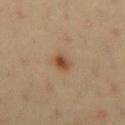No biopsy was performed on this lesion — it was imaged during a full skin examination and was not determined to be concerning. Imaged with cross-polarized lighting. A region of skin cropped from a whole-body photographic capture, roughly 15 mm wide. Automated image analysis of the tile measured a border-irregularity rating of about 2.5/10 and a peripheral color-asymmetry measure near 2. The software also gave lesion-presence confidence of about 100/100. About 2.5 mm across. From the chest. A male patient aged 38–42.